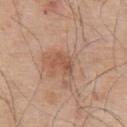Q: How was the tile lit?
A: white-light illumination
Q: Lesion location?
A: the upper back
Q: Patient demographics?
A: male, in their mid-50s
Q: How was this image acquired?
A: 15 mm crop, total-body photography